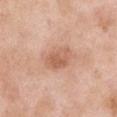<case>
<biopsy_status>not biopsied; imaged during a skin examination</biopsy_status>
<lighting>white-light</lighting>
<site>right upper arm</site>
<image>
  <source>total-body photography crop</source>
  <field_of_view_mm>15</field_of_view_mm>
</image>
<lesion_size>
  <long_diameter_mm_approx>4.0</long_diameter_mm_approx>
</lesion_size>
<patient>
  <sex>male</sex>
  <age_approx>55</age_approx>
</patient>
<automated_metrics>
  <area_mm2_approx>7.5</area_mm2_approx>
  <eccentricity>0.75</eccentricity>
  <shape_asymmetry>0.25</shape_asymmetry>
</automated_metrics>
</case>